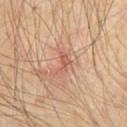| feature | finding |
|---|---|
| workup | no biopsy performed (imaged during a skin exam) |
| imaging modality | ~15 mm crop, total-body skin-cancer survey |
| lesion diameter | ≈3 mm |
| site | the abdomen |
| subject | male, aged 68 to 72 |
| lighting | cross-polarized |